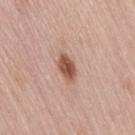A female patient aged 63 to 67.
The lesion is on the leg.
A lesion tile, about 15 mm wide, cut from a 3D total-body photograph.